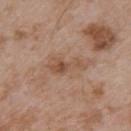Case summary:
• notes: catalogued during a skin exam; not biopsied
• body site: the upper back
• lesion size: ≈4.5 mm
• acquisition: total-body-photography crop, ~15 mm field of view
• lighting: white-light
• subject: male, aged 53 to 57